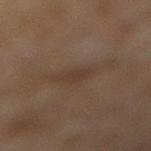| key | value |
|---|---|
| follow-up | no biopsy performed (imaged during a skin exam) |
| lesion diameter | ~2.5 mm (longest diameter) |
| automated lesion analysis | an area of roughly 3.5 mm², an eccentricity of roughly 0.75, and a symmetry-axis asymmetry near 0.3; roughly 5 lightness units darker than nearby skin; a border-irregularity rating of about 3/10, internal color variation of about 1 on a 0–10 scale, and peripheral color asymmetry of about 0.5; a nevus-likeness score of about 5/100 and lesion-presence confidence of about 100/100 |
| anatomic site | the right lower leg |
| tile lighting | cross-polarized illumination |
| subject | female, aged 58 to 62 |
| image | 15 mm crop, total-body photography |